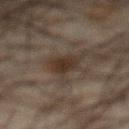The lesion was photographed on a routine skin check and not biopsied; there is no pathology result. Approximately 3.5 mm at its widest. A close-up tile cropped from a whole-body skin photograph, about 15 mm across. Captured under cross-polarized illumination. Automated image analysis of the tile measured a classifier nevus-likeness of about 90/100 and lesion-presence confidence of about 100/100. The lesion is on the abdomen. A male subject about 65 years old.